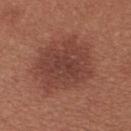workup: catalogued during a skin exam; not biopsied
subject: female, aged 23 to 27
image: total-body-photography crop, ~15 mm field of view
site: the upper back
lesion diameter: ≈6.5 mm